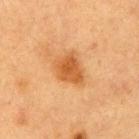{
  "biopsy_status": "not biopsied; imaged during a skin examination",
  "site": "right upper arm",
  "image": {
    "source": "total-body photography crop",
    "field_of_view_mm": 15
  },
  "patient": {
    "sex": "female",
    "age_approx": 40
  },
  "lesion_size": {
    "long_diameter_mm_approx": 4.0
  },
  "lighting": "cross-polarized"
}